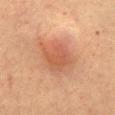Findings:
• notes — catalogued during a skin exam; not biopsied
• subject — female, aged around 60
• diameter — ~4 mm (longest diameter)
• tile lighting — cross-polarized illumination
• body site — the abdomen
• acquisition — total-body-photography crop, ~15 mm field of view